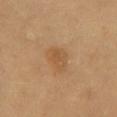Notes:
* workup — no biopsy performed (imaged during a skin exam)
* site — the chest
* image — 15 mm crop, total-body photography
* patient — female, in their mid-50s
* diameter — ~3 mm (longest diameter)
* image-analysis metrics — a lesion-detection confidence of about 100/100